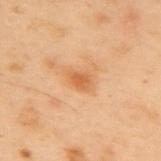| field | value |
|---|---|
| notes | imaged on a skin check; not biopsied |
| lesion size | ≈2.5 mm |
| imaging modality | ~15 mm tile from a whole-body skin photo |
| anatomic site | the mid back |
| tile lighting | cross-polarized illumination |
| patient | male, aged around 55 |